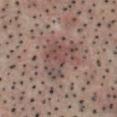Assessment:
Imaged during a routine full-body skin examination; the lesion was not biopsied and no histopathology is available.
Background:
Captured under cross-polarized illumination. The lesion is located on the head or neck. A male subject aged approximately 55. A lesion tile, about 15 mm wide, cut from a 3D total-body photograph. Longest diameter approximately 5.5 mm. The total-body-photography lesion software estimated an average lesion color of about L≈45 a*≈15 b*≈19 (CIELAB), roughly 8 lightness units darker than nearby skin, and a normalized border contrast of about 6.5. It also reported a within-lesion color-variation index near 5.5/10 and a peripheral color-asymmetry measure near 2.